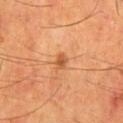<record>
<biopsy_status>not biopsied; imaged during a skin examination</biopsy_status>
<patient>
  <sex>male</sex>
  <age_approx>60</age_approx>
</patient>
<site>mid back</site>
<automated_metrics>
  <area_mm2_approx>2.5</area_mm2_approx>
  <shape_asymmetry>0.2</shape_asymmetry>
  <cielab_L>53</cielab_L>
  <cielab_a>26</cielab_a>
  <cielab_b>41</cielab_b>
  <vs_skin_darker_L>10.0</vs_skin_darker_L>
  <vs_skin_contrast_norm>7.0</vs_skin_contrast_norm>
  <nevus_likeness_0_100>80</nevus_likeness_0_100>
  <lesion_detection_confidence_0_100>100</lesion_detection_confidence_0_100>
</automated_metrics>
<image>
  <source>total-body photography crop</source>
  <field_of_view_mm>15</field_of_view_mm>
</image>
<lesion_size>
  <long_diameter_mm_approx>2.0</long_diameter_mm_approx>
</lesion_size>
</record>